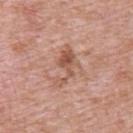  biopsy_status: not biopsied; imaged during a skin examination
  automated_metrics:
    cielab_L: 55
    cielab_a: 22
    cielab_b: 29
    vs_skin_contrast_norm: 6.5
    border_irregularity_0_10: 7.0
    color_variation_0_10: 4.5
    peripheral_color_asymmetry: 1.5
    lesion_detection_confidence_0_100: 100
  patient:
    sex: male
    age_approx: 50
  site: back
  image:
    source: total-body photography crop
    field_of_view_mm: 15